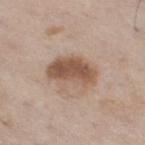Assessment: Imaged during a routine full-body skin examination; the lesion was not biopsied and no histopathology is available.